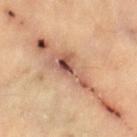Q: Was a biopsy performed?
A: imaged on a skin check; not biopsied
Q: What are the patient's age and sex?
A: approximately 60 years of age
Q: What lighting was used for the tile?
A: cross-polarized illumination
Q: Automated lesion metrics?
A: an area of roughly 9 mm², a shape eccentricity near 0.95, and two-axis asymmetry of about 0.5; about 14 CIELAB-L* units darker than the surrounding skin and a normalized lesion–skin contrast near 9.5; an automated nevus-likeness rating near 0 out of 100 and a detector confidence of about 60 out of 100 that the crop contains a lesion
Q: What is the imaging modality?
A: ~15 mm tile from a whole-body skin photo
Q: Lesion location?
A: the right lower leg
Q: What is the lesion's diameter?
A: about 6 mm